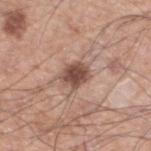Notes:
- workup — imaged on a skin check; not biopsied
- image — ~15 mm tile from a whole-body skin photo
- automated metrics — a footprint of about 7 mm², an eccentricity of roughly 0.65, and a symmetry-axis asymmetry near 0.25; an average lesion color of about L≈49 a*≈20 b*≈25 (CIELAB) and a lesion-to-skin contrast of about 10 (normalized; higher = more distinct); a border-irregularity rating of about 2.5/10 and radial color variation of about 1; a nevus-likeness score of about 95/100 and a lesion-detection confidence of about 100/100
- location — the left lower leg
- subject — male, aged 58 to 62
- diameter — ≈3.5 mm
- tile lighting — white-light